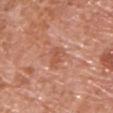Findings:
* patient · male, aged 63 to 67
* TBP lesion metrics · border irregularity of about 3.5 on a 0–10 scale, a color-variation rating of about 2.5/10, and peripheral color asymmetry of about 1
* location · the chest
* size · ~3 mm (longest diameter)
* image source · 15 mm crop, total-body photography
* illumination · white-light